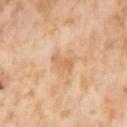Context:
A female subject, aged 53–57. The lesion is on the right thigh. Imaged with cross-polarized lighting. A 15 mm crop from a total-body photograph taken for skin-cancer surveillance.